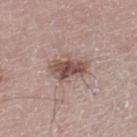biopsy status=no biopsy performed (imaged during a skin exam) | tile lighting=white-light | acquisition=~15 mm tile from a whole-body skin photo | subject=male, in their mid-60s | lesion diameter=≈4.5 mm | anatomic site=the left thigh.